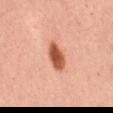biopsy status=imaged on a skin check; not biopsied